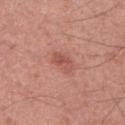The lesion was tiled from a total-body skin photograph and was not biopsied.
Located on the arm.
A male subject, aged approximately 35.
The tile uses white-light illumination.
A roughly 15 mm field-of-view crop from a total-body skin photograph.